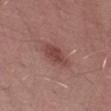  biopsy_status: not biopsied; imaged during a skin examination
  patient:
    sex: male
    age_approx: 50
  lesion_size:
    long_diameter_mm_approx: 4.5
  image:
    source: total-body photography crop
    field_of_view_mm: 15
  site: left thigh